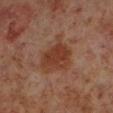lighting: cross-polarized illumination | lesion diameter: ≈5 mm | body site: the left lower leg | patient: male, aged around 60 | image source: 15 mm crop, total-body photography.